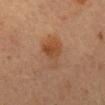Q: What did automated image analysis measure?
A: an average lesion color of about L≈41 a*≈19 b*≈32 (CIELAB), roughly 7 lightness units darker than nearby skin, and a normalized lesion–skin contrast near 7
Q: What is the lesion's diameter?
A: ≈4.5 mm
Q: Illumination type?
A: cross-polarized illumination
Q: What are the patient's age and sex?
A: female, aged 58 to 62
Q: Where on the body is the lesion?
A: the mid back
Q: How was this image acquired?
A: 15 mm crop, total-body photography